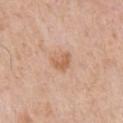biopsy status: no biopsy performed (imaged during a skin exam) | subject: male, about 80 years old | site: the chest | lesion size: about 3 mm | image source: ~15 mm tile from a whole-body skin photo.